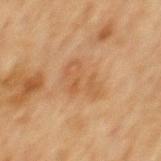workup: total-body-photography surveillance lesion; no biopsy
image source: 15 mm crop, total-body photography
patient: male, aged around 65
body site: the back